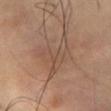Assessment: The lesion was tiled from a total-body skin photograph and was not biopsied. Image and clinical context: A male subject, about 55 years old. A close-up tile cropped from a whole-body skin photograph, about 15 mm across. The lesion is located on the abdomen. An algorithmic analysis of the crop reported a footprint of about 14 mm², a shape eccentricity near 0.75, and two-axis asymmetry of about 0.45. And it measured an average lesion color of about L≈47 a*≈17 b*≈27 (CIELAB), about 6 CIELAB-L* units darker than the surrounding skin, and a normalized lesion–skin contrast near 5.